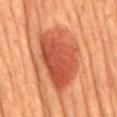- workup · imaged on a skin check; not biopsied
- TBP lesion metrics · an area of roughly 32 mm²; a lesion–skin lightness drop of about 13 and a normalized border contrast of about 9; a border-irregularity rating of about 3.5/10; a nevus-likeness score of about 100/100 and lesion-presence confidence of about 100/100
- lighting · cross-polarized
- lesion size · about 9 mm
- imaging modality · 15 mm crop, total-body photography
- site · the front of the torso
- patient · male, aged 63 to 67